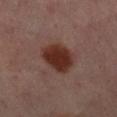| field | value |
|---|---|
| biopsy status | no biopsy performed (imaged during a skin exam) |
| location | the leg |
| tile lighting | cross-polarized illumination |
| automated lesion analysis | a border-irregularity index near 1.5/10 and internal color variation of about 3 on a 0–10 scale |
| patient | female, aged 48–52 |
| acquisition | total-body-photography crop, ~15 mm field of view |
| size | ≈4 mm |